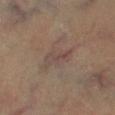Recorded during total-body skin imaging; not selected for excision or biopsy. A lesion tile, about 15 mm wide, cut from a 3D total-body photograph. Captured under cross-polarized illumination. Measured at roughly 4 mm in maximum diameter. A male patient aged approximately 60. Located on the right lower leg. An algorithmic analysis of the crop reported a lesion-to-skin contrast of about 5.5 (normalized; higher = more distinct).